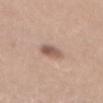Findings:
- biopsy status · imaged on a skin check; not biopsied
- lesion size · ≈3 mm
- subject · male, roughly 55 years of age
- location · the mid back
- acquisition · ~15 mm crop, total-body skin-cancer survey